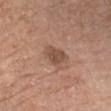| feature | finding |
|---|---|
| biopsy status | total-body-photography surveillance lesion; no biopsy |
| site | the chest |
| image | total-body-photography crop, ~15 mm field of view |
| subject | male, aged around 80 |
| illumination | white-light |
| image-analysis metrics | an average lesion color of about L≈50 a*≈19 b*≈28 (CIELAB), about 9 CIELAB-L* units darker than the surrounding skin, and a lesion-to-skin contrast of about 7 (normalized; higher = more distinct); a border-irregularity rating of about 2.5/10, internal color variation of about 2.5 on a 0–10 scale, and a peripheral color-asymmetry measure near 1; an automated nevus-likeness rating near 5 out of 100 and a detector confidence of about 100 out of 100 that the crop contains a lesion |